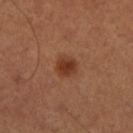Impression:
The lesion was tiled from a total-body skin photograph and was not biopsied.
Clinical summary:
The subject is a male aged approximately 50. This is a cross-polarized tile. A 15 mm crop from a total-body photograph taken for skin-cancer surveillance. Located on the leg.